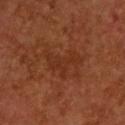Q: Was this lesion biopsied?
A: total-body-photography surveillance lesion; no biopsy
Q: How was this image acquired?
A: ~15 mm tile from a whole-body skin photo
Q: What are the patient's age and sex?
A: male, about 55 years old
Q: Where on the body is the lesion?
A: the upper back